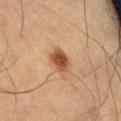Q: Was a biopsy performed?
A: no biopsy performed (imaged during a skin exam)
Q: What is the anatomic site?
A: the leg
Q: What is the imaging modality?
A: ~15 mm crop, total-body skin-cancer survey
Q: What are the patient's age and sex?
A: male, in their mid-60s
Q: What is the lesion's diameter?
A: ≈3.5 mm
Q: Illumination type?
A: cross-polarized